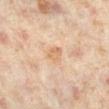workup: total-body-photography surveillance lesion; no biopsy
lighting: cross-polarized
image-analysis metrics: an eccentricity of roughly 0.75 and a shape-asymmetry score of about 0.25 (0 = symmetric); a border-irregularity index near 2/10, internal color variation of about 3 on a 0–10 scale, and peripheral color asymmetry of about 1; a lesion-detection confidence of about 100/100
subject: female, about 50 years old
anatomic site: the right lower leg
image: total-body-photography crop, ~15 mm field of view
lesion diameter: ≈2.5 mm